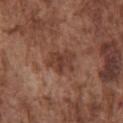Imaged during a routine full-body skin examination; the lesion was not biopsied and no histopathology is available.
Measured at roughly 4 mm in maximum diameter.
The lesion is on the chest.
Cropped from a total-body skin-imaging series; the visible field is about 15 mm.
A male patient, in their mid- to late 70s.
Imaged with white-light lighting.